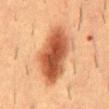follow-up: no biopsy performed (imaged during a skin exam); location: the mid back; subject: male, about 55 years old; image source: ~15 mm tile from a whole-body skin photo.